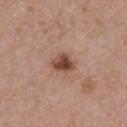subject = female, aged approximately 30
imaging modality = ~15 mm tile from a whole-body skin photo
illumination = white-light illumination
location = the upper back
TBP lesion metrics = a border-irregularity rating of about 2/10, a within-lesion color-variation index near 4.5/10, and peripheral color asymmetry of about 1.5